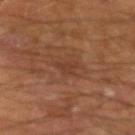  site: left forearm
  image:
    source: total-body photography crop
    field_of_view_mm: 15
  lighting: cross-polarized
  lesion_size:
    long_diameter_mm_approx: 2.5
  patient:
    sex: male
    age_approx: 65
  automated_metrics:
    cielab_L: 36
    cielab_a: 21
    cielab_b: 28
    vs_skin_darker_L: 5.0
    vs_skin_contrast_norm: 4.5
    border_irregularity_0_10: 4.0
    color_variation_0_10: 1.0
    nevus_likeness_0_100: 0
    lesion_detection_confidence_0_100: 100